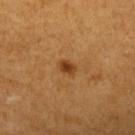The lesion was tiled from a total-body skin photograph and was not biopsied. The patient is a female aged 53 to 57. An algorithmic analysis of the crop reported a border-irregularity index near 2.5/10 and a peripheral color-asymmetry measure near 0.5. The software also gave a nevus-likeness score of about 100/100 and a detector confidence of about 100 out of 100 that the crop contains a lesion. Longest diameter approximately 2 mm. Located on the arm. Cropped from a whole-body photographic skin survey; the tile spans about 15 mm.